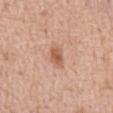Q: Was a biopsy performed?
A: catalogued during a skin exam; not biopsied
Q: What is the lesion's diameter?
A: about 2.5 mm
Q: What is the imaging modality?
A: 15 mm crop, total-body photography
Q: What are the patient's age and sex?
A: male, approximately 60 years of age
Q: Lesion location?
A: the abdomen